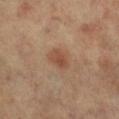{"biopsy_status": "not biopsied; imaged during a skin examination", "lesion_size": {"long_diameter_mm_approx": 3.0}, "image": {"source": "total-body photography crop", "field_of_view_mm": 15}, "automated_metrics": {"area_mm2_approx": 5.5, "eccentricity": 0.7, "cielab_L": 47, "cielab_a": 20, "cielab_b": 30, "vs_skin_darker_L": 8.0, "vs_skin_contrast_norm": 6.0, "lesion_detection_confidence_0_100": 100}, "site": "left leg", "lighting": "cross-polarized", "patient": {"sex": "female", "age_approx": 65}}